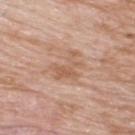The lesion was tiled from a total-body skin photograph and was not biopsied.
From the upper back.
A close-up tile cropped from a whole-body skin photograph, about 15 mm across.
A male subject, roughly 60 years of age.